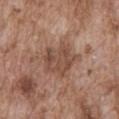* follow-up: total-body-photography surveillance lesion; no biopsy
* image: ~15 mm tile from a whole-body skin photo
* lesion diameter: ~4.5 mm (longest diameter)
* subject: male, roughly 75 years of age
* body site: the abdomen
* automated lesion analysis: a mean CIELAB color near L≈48 a*≈19 b*≈27, roughly 9 lightness units darker than nearby skin, and a normalized lesion–skin contrast near 6.5; border irregularity of about 3.5 on a 0–10 scale, a within-lesion color-variation index near 3.5/10, and a peripheral color-asymmetry measure near 1